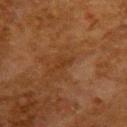{"biopsy_status": "not biopsied; imaged during a skin examination", "image": {"source": "total-body photography crop", "field_of_view_mm": 15}, "lesion_size": {"long_diameter_mm_approx": 2.5}, "lighting": "cross-polarized", "site": "upper back", "patient": {"sex": "female", "age_approx": 50}}